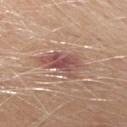No biopsy was performed on this lesion — it was imaged during a full skin examination and was not determined to be concerning.
A region of skin cropped from a whole-body photographic capture, roughly 15 mm wide.
Captured under white-light illumination.
The patient is a male aged around 65.
From the chest.